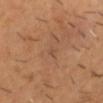Imaged during a routine full-body skin examination; the lesion was not biopsied and no histopathology is available.